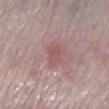biopsy status = catalogued during a skin exam; not biopsied | diameter = ≈3 mm | location = the left lower leg | patient = male, aged approximately 65 | image source = ~15 mm crop, total-body skin-cancer survey | lighting = white-light | automated lesion analysis = an average lesion color of about L≈54 a*≈24 b*≈20 (CIELAB) and a lesion–skin lightness drop of about 7; border irregularity of about 3.5 on a 0–10 scale, a within-lesion color-variation index near 1.5/10, and radial color variation of about 0.5.